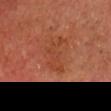Impression:
Imaged during a routine full-body skin examination; the lesion was not biopsied and no histopathology is available.
Context:
The subject is a male approximately 65 years of age. The tile uses cross-polarized illumination. Cropped from a total-body skin-imaging series; the visible field is about 15 mm. The lesion is located on the head or neck.